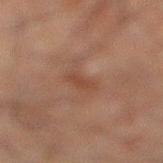Clinical summary:
A 15 mm close-up extracted from a 3D total-body photography capture. Captured under cross-polarized illumination. From the left leg. The subject is a male aged 58 to 62. Measured at roughly 3 mm in maximum diameter. Automated image analysis of the tile measured a lesion color around L≈33 a*≈17 b*≈23 in CIELAB, a lesion–skin lightness drop of about 5, and a normalized border contrast of about 5.5.